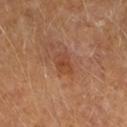Case summary:
– notes · no biopsy performed (imaged during a skin exam)
– size · ~3 mm (longest diameter)
– image source · ~15 mm tile from a whole-body skin photo
– location · the right forearm
– subject · female, approximately 60 years of age